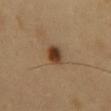The lesion was photographed on a routine skin check and not biopsied; there is no pathology result. A 15 mm close-up tile from a total-body photography series done for melanoma screening. The subject is a male roughly 50 years of age. Captured under cross-polarized illumination. On the front of the torso. The recorded lesion diameter is about 2.5 mm.